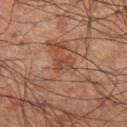| feature | finding |
|---|---|
| follow-up | no biopsy performed (imaged during a skin exam) |
| anatomic site | the left thigh |
| tile lighting | cross-polarized |
| automated lesion analysis | an average lesion color of about L≈44 a*≈23 b*≈30 (CIELAB), roughly 7 lightness units darker than nearby skin, and a lesion-to-skin contrast of about 5.5 (normalized; higher = more distinct); a border-irregularity index near 3.5/10, a within-lesion color-variation index near 2.5/10, and peripheral color asymmetry of about 1 |
| size | about 2 mm |
| subject | male, in their 60s |
| acquisition | ~15 mm crop, total-body skin-cancer survey |